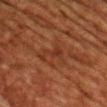Q: Is there a histopathology result?
A: total-body-photography surveillance lesion; no biopsy
Q: Who is the patient?
A: male, aged 58–62
Q: What did automated image analysis measure?
A: a border-irregularity index near 6.5/10, internal color variation of about 1.5 on a 0–10 scale, and peripheral color asymmetry of about 0.5; a nevus-likeness score of about 0/100 and lesion-presence confidence of about 90/100
Q: What is the lesion's diameter?
A: about 3.5 mm
Q: Where on the body is the lesion?
A: the chest
Q: How was this image acquired?
A: 15 mm crop, total-body photography
Q: Illumination type?
A: cross-polarized